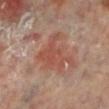The lesion was tiled from a total-body skin photograph and was not biopsied. About 5 mm across. Located on the leg. Cropped from a whole-body photographic skin survey; the tile spans about 15 mm. The tile uses cross-polarized illumination. The patient is a female about 60 years old.